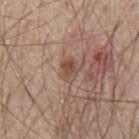follow-up — imaged on a skin check; not biopsied | image — ~15 mm tile from a whole-body skin photo | patient — male, aged 63 to 67 | anatomic site — the mid back | automated lesion analysis — an area of roughly 4 mm², a shape eccentricity near 0.75, and a shape-asymmetry score of about 0.25 (0 = symmetric); border irregularity of about 2.5 on a 0–10 scale, internal color variation of about 5 on a 0–10 scale, and radial color variation of about 2; an automated nevus-likeness rating near 10 out of 100 and lesion-presence confidence of about 100/100.